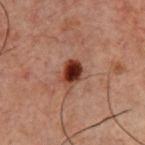The subject is a male aged 58 to 62.
A region of skin cropped from a whole-body photographic capture, roughly 15 mm wide.
Longest diameter approximately 3 mm.
The lesion is located on the chest.
Captured under cross-polarized illumination.